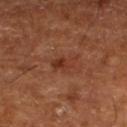notes: catalogued during a skin exam; not biopsied, subject: in their mid- to late 60s, lighting: cross-polarized illumination, diameter: about 3 mm, location: the left lower leg, image source: ~15 mm tile from a whole-body skin photo.